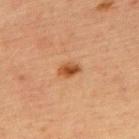Imaged during a routine full-body skin examination; the lesion was not biopsied and no histopathology is available. Located on the upper back. Cropped from a whole-body photographic skin survey; the tile spans about 15 mm. A female patient, in their mid-60s.